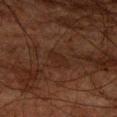<record>
  <biopsy_status>not biopsied; imaged during a skin examination</biopsy_status>
  <patient>
    <sex>male</sex>
    <age_approx>80</age_approx>
  </patient>
  <lesion_size>
    <long_diameter_mm_approx>2.5</long_diameter_mm_approx>
  </lesion_size>
  <automated_metrics>
    <border_irregularity_0_10>2.5</border_irregularity_0_10>
    <peripheral_color_asymmetry>0.0</peripheral_color_asymmetry>
  </automated_metrics>
  <image>
    <source>total-body photography crop</source>
    <field_of_view_mm>15</field_of_view_mm>
  </image>
  <site>left forearm</site>
</record>